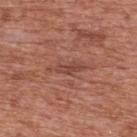{"biopsy_status": "not biopsied; imaged during a skin examination", "lighting": "white-light", "lesion_size": {"long_diameter_mm_approx": 4.0}, "patient": {"sex": "male", "age_approx": 70}, "automated_metrics": {"border_irregularity_0_10": 4.5, "peripheral_color_asymmetry": 0.5, "nevus_likeness_0_100": 0}, "site": "upper back", "image": {"source": "total-body photography crop", "field_of_view_mm": 15}}